A lesion tile, about 15 mm wide, cut from a 3D total-body photograph. A female patient, approximately 45 years of age. The lesion is located on the mid back.
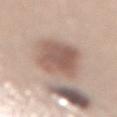Image and clinical context:
About 7 mm across. The total-body-photography lesion software estimated a mean CIELAB color near L≈57 a*≈17 b*≈25, about 12 CIELAB-L* units darker than the surrounding skin, and a normalized lesion–skin contrast near 8. This is a white-light tile.
Diagnosis:
The lesion was biopsied, and histopathology showed an atypical melanocytic neoplasm — an indeterminate (borderline) lesion.